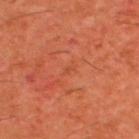Part of a total-body skin-imaging series; this lesion was reviewed on a skin check and was not flagged for biopsy. Cropped from a whole-body photographic skin survey; the tile spans about 15 mm. The patient is a male in their 60s. On the upper back.